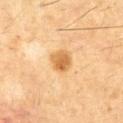Clinical impression:
Recorded during total-body skin imaging; not selected for excision or biopsy.
Background:
A male subject, aged 58–62. Captured under cross-polarized illumination. Measured at roughly 2.5 mm in maximum diameter. The lesion is located on the chest. Automated tile analysis of the lesion measured a lesion area of about 6 mm² and an outline eccentricity of about 0.2 (0 = round, 1 = elongated). The software also gave a border-irregularity rating of about 1.5/10, a within-lesion color-variation index near 4/10, and radial color variation of about 1.5. The software also gave a classifier nevus-likeness of about 80/100 and a detector confidence of about 100 out of 100 that the crop contains a lesion. A 15 mm close-up tile from a total-body photography series done for melanoma screening.